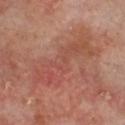A 15 mm crop from a total-body photograph taken for skin-cancer surveillance.
A male patient, aged 63–67.
The lesion is on the head or neck.
This is a cross-polarized tile.
The recorded lesion diameter is about 9.5 mm.
The total-body-photography lesion software estimated a lesion color around L≈47 a*≈25 b*≈27 in CIELAB, a lesion–skin lightness drop of about 6, and a lesion-to-skin contrast of about 5 (normalized; higher = more distinct). And it measured an automated nevus-likeness rating near 0 out of 100 and lesion-presence confidence of about 100/100.